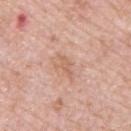  biopsy_status: not biopsied; imaged during a skin examination
  image:
    source: total-body photography crop
    field_of_view_mm: 15
  site: upper back
  lighting: white-light
  patient:
    sex: female
    age_approx: 45